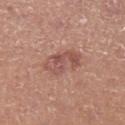Part of a total-body skin-imaging series; this lesion was reviewed on a skin check and was not flagged for biopsy. A male subject about 55 years old. Approximately 5 mm at its widest. A close-up tile cropped from a whole-body skin photograph, about 15 mm across. Automated tile analysis of the lesion measured an outline eccentricity of about 0.85 (0 = round, 1 = elongated). And it measured a border-irregularity rating of about 3/10, a color-variation rating of about 5/10, and a peripheral color-asymmetry measure near 2. Imaged with white-light lighting. The lesion is located on the left lower leg.